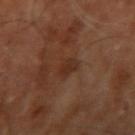Assessment: Part of a total-body skin-imaging series; this lesion was reviewed on a skin check and was not flagged for biopsy. Clinical summary: A male subject, aged approximately 70. A 15 mm close-up tile from a total-body photography series done for melanoma screening. Located on the right upper arm.